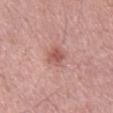Q: Was this lesion biopsied?
A: no biopsy performed (imaged during a skin exam)
Q: Lesion location?
A: the mid back
Q: Automated lesion metrics?
A: a mean CIELAB color near L≈55 a*≈26 b*≈25, roughly 10 lightness units darker than nearby skin, and a normalized lesion–skin contrast near 7
Q: Who is the patient?
A: male, aged around 60
Q: What lighting was used for the tile?
A: white-light
Q: Lesion size?
A: ≈2.5 mm
Q: What kind of image is this?
A: total-body-photography crop, ~15 mm field of view